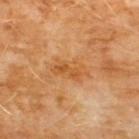notes = catalogued during a skin exam; not biopsied
lesion diameter = ≈4.5 mm
automated metrics = a mean CIELAB color near L≈53 a*≈24 b*≈43, about 7 CIELAB-L* units darker than the surrounding skin, and a normalized border contrast of about 6
imaging modality = ~15 mm crop, total-body skin-cancer survey
site = the chest
patient = male, aged 58–62